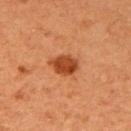Part of a total-body skin-imaging series; this lesion was reviewed on a skin check and was not flagged for biopsy. A 15 mm close-up extracted from a 3D total-body photography capture. On the right upper arm. Longest diameter approximately 3.5 mm. A female subject, aged approximately 40.